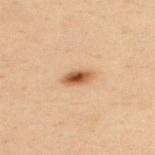Findings:
* workup · no biopsy performed (imaged during a skin exam)
* image source · ~15 mm tile from a whole-body skin photo
* tile lighting · cross-polarized
* automated metrics · a lesion area of about 4.5 mm², an eccentricity of roughly 0.85, and two-axis asymmetry of about 0.2; an average lesion color of about L≈46 a*≈19 b*≈31 (CIELAB) and roughly 13 lightness units darker than nearby skin; a border-irregularity rating of about 1.5/10, a color-variation rating of about 6/10, and a peripheral color-asymmetry measure near 1.5
* lesion size · about 3 mm
* patient · male, aged 28–32
* location · the upper back